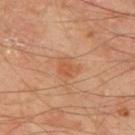workup = total-body-photography surveillance lesion; no biopsy
body site = the mid back
image = total-body-photography crop, ~15 mm field of view
patient = male, approximately 65 years of age
TBP lesion metrics = a nevus-likeness score of about 10/100